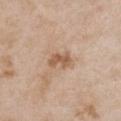workup=no biopsy performed (imaged during a skin exam) | acquisition=total-body-photography crop, ~15 mm field of view | patient=female, aged around 30 | location=the front of the torso | size=about 3 mm | tile lighting=white-light illumination.